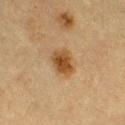Part of a total-body skin-imaging series; this lesion was reviewed on a skin check and was not flagged for biopsy. A lesion tile, about 15 mm wide, cut from a 3D total-body photograph. The recorded lesion diameter is about 3.5 mm. On the right thigh. A female patient aged approximately 55. Captured under cross-polarized illumination. The lesion-visualizer software estimated a lesion area of about 7.5 mm², an outline eccentricity of about 0.55 (0 = round, 1 = elongated), and a symmetry-axis asymmetry near 0.2. It also reported a border-irregularity index near 2/10, a within-lesion color-variation index near 4/10, and a peripheral color-asymmetry measure near 1. The software also gave a nevus-likeness score of about 100/100 and lesion-presence confidence of about 100/100.